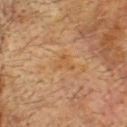follow-up — no biopsy performed (imaged during a skin exam)
subject — male, aged approximately 75
illumination — cross-polarized
acquisition — 15 mm crop, total-body photography
lesion diameter — ~2.5 mm (longest diameter)
location — the head or neck